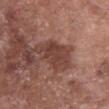Q: Is there a histopathology result?
A: catalogued during a skin exam; not biopsied
Q: What is the imaging modality?
A: total-body-photography crop, ~15 mm field of view
Q: What are the patient's age and sex?
A: female, aged around 75
Q: Lesion location?
A: the chest
Q: How large is the lesion?
A: ≈5 mm
Q: What lighting was used for the tile?
A: white-light illumination